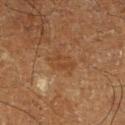biopsy status: no biopsy performed (imaged during a skin exam) | subject: male, approximately 65 years of age | automated metrics: an area of roughly 5.5 mm², an eccentricity of roughly 0.7, and a shape-asymmetry score of about 0.3 (0 = symmetric); a classifier nevus-likeness of about 0/100 | lesion size: ≈3 mm | imaging modality: total-body-photography crop, ~15 mm field of view | location: the right lower leg.